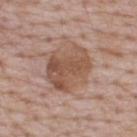{
  "biopsy_status": "not biopsied; imaged during a skin examination",
  "image": {
    "source": "total-body photography crop",
    "field_of_view_mm": 15
  },
  "patient": {
    "sex": "male",
    "age_approx": 65
  },
  "site": "upper back",
  "lesion_size": {
    "long_diameter_mm_approx": 5.5
  },
  "lighting": "white-light",
  "automated_metrics": {
    "border_irregularity_0_10": 2.0,
    "color_variation_0_10": 5.0,
    "nevus_likeness_0_100": 25,
    "lesion_detection_confidence_0_100": 100
  }
}